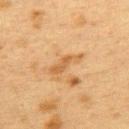Q: Was this lesion biopsied?
A: catalogued during a skin exam; not biopsied
Q: Patient demographics?
A: female, approximately 40 years of age
Q: Automated lesion metrics?
A: a nevus-likeness score of about 0/100
Q: Where on the body is the lesion?
A: the upper back
Q: How was this image acquired?
A: 15 mm crop, total-body photography
Q: What lighting was used for the tile?
A: cross-polarized illumination